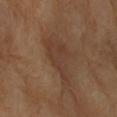Part of a total-body skin-imaging series; this lesion was reviewed on a skin check and was not flagged for biopsy. A female subject aged around 70. A 15 mm close-up tile from a total-body photography series done for melanoma screening. The total-body-photography lesion software estimated a lesion area of about 11 mm². The software also gave a border-irregularity index near 6.5/10, internal color variation of about 2.5 on a 0–10 scale, and peripheral color asymmetry of about 0.5. The recorded lesion diameter is about 6.5 mm. This is a cross-polarized tile. The lesion is located on the left forearm.